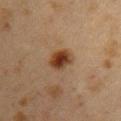The lesion was tiled from a total-body skin photograph and was not biopsied. The lesion is located on the right upper arm. Captured under cross-polarized illumination. The total-body-photography lesion software estimated an area of roughly 6.5 mm², a shape eccentricity near 0.6, and two-axis asymmetry of about 0.2. It also reported a lesion color around L≈33 a*≈17 b*≈28 in CIELAB. The lesion's longest dimension is about 3 mm. A female subject, aged 38 to 42. Cropped from a total-body skin-imaging series; the visible field is about 15 mm.